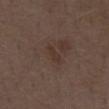Captured during whole-body skin photography for melanoma surveillance; the lesion was not biopsied. The lesion is located on the abdomen. The tile uses white-light illumination. A male subject aged around 70. A 15 mm close-up tile from a total-body photography series done for melanoma screening.